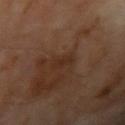| field | value |
|---|---|
| subject | male, aged around 70 |
| acquisition | total-body-photography crop, ~15 mm field of view |
| tile lighting | cross-polarized illumination |
| anatomic site | the right upper arm |